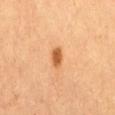No biopsy was performed on this lesion — it was imaged during a full skin examination and was not determined to be concerning. A 15 mm close-up tile from a total-body photography series done for melanoma screening. From the right thigh. Measured at roughly 2.5 mm in maximum diameter. A female subject aged 68–72.